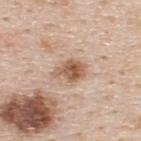This lesion was catalogued during total-body skin photography and was not selected for biopsy.
The lesion is located on the upper back.
Automated tile analysis of the lesion measured a lesion area of about 7 mm² and an outline eccentricity of about 0.75 (0 = round, 1 = elongated). The analysis additionally found a detector confidence of about 100 out of 100 that the crop contains a lesion.
A male patient, aged 43–47.
A roughly 15 mm field-of-view crop from a total-body skin photograph.
Longest diameter approximately 4 mm.